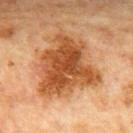Automated tile analysis of the lesion measured a footprint of about 34 mm² and an outline eccentricity of about 0.45 (0 = round, 1 = elongated). It also reported a within-lesion color-variation index near 5.5/10. This image is a 15 mm lesion crop taken from a total-body photograph. Captured under cross-polarized illumination. The lesion is on the mid back. A male subject, roughly 65 years of age.